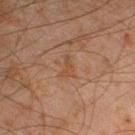Q: Was this lesion biopsied?
A: catalogued during a skin exam; not biopsied
Q: Lesion size?
A: about 2.5 mm
Q: How was the tile lit?
A: cross-polarized
Q: Patient demographics?
A: male, about 45 years old
Q: How was this image acquired?
A: ~15 mm tile from a whole-body skin photo
Q: Lesion location?
A: the left lower leg